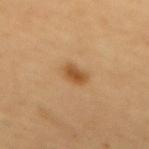| field | value |
|---|---|
| follow-up | imaged on a skin check; not biopsied |
| lesion diameter | ~3 mm (longest diameter) |
| image source | 15 mm crop, total-body photography |
| illumination | cross-polarized |
| automated lesion analysis | a footprint of about 4.5 mm² and an outline eccentricity of about 0.8 (0 = round, 1 = elongated) |
| subject | female, in their mid-50s |
| body site | the back |